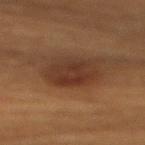Case summary:
- workup: catalogued during a skin exam; not biopsied
- tile lighting: cross-polarized illumination
- site: the chest
- lesion size: ≈5.5 mm
- image source: total-body-photography crop, ~15 mm field of view
- patient: female, roughly 80 years of age
- automated metrics: roughly 7 lightness units darker than nearby skin and a normalized lesion–skin contrast near 8.5